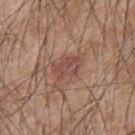Captured during whole-body skin photography for melanoma surveillance; the lesion was not biopsied.
A male subject roughly 45 years of age.
Cropped from a total-body skin-imaging series; the visible field is about 15 mm.
The lesion is on the upper back.